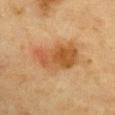acquisition = ~15 mm crop, total-body skin-cancer survey
patient = female, in their mid-50s
illumination = cross-polarized
location = the chest